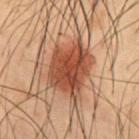Recorded during total-body skin imaging; not selected for excision or biopsy.
Automated tile analysis of the lesion measured an eccentricity of roughly 0.6 and two-axis asymmetry of about 0.25. It also reported a mean CIELAB color near L≈39 a*≈22 b*≈28, a lesion–skin lightness drop of about 13, and a normalized border contrast of about 10.5.
The lesion is on the front of the torso.
Cropped from a total-body skin-imaging series; the visible field is about 15 mm.
Longest diameter approximately 6 mm.
The patient is a male roughly 55 years of age.